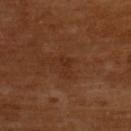  patient:
    sex: female
    age_approx: 55
  automated_metrics:
    eccentricity: 0.85
    shape_asymmetry: 0.5
    color_variation_0_10: 0.5
    peripheral_color_asymmetry: 0.0
    nevus_likeness_0_100: 0
    lesion_detection_confidence_0_100: 100
  lesion_size:
    long_diameter_mm_approx: 2.5
  image:
    source: total-body photography crop
    field_of_view_mm: 15
  site: upper back
  lighting: cross-polarized